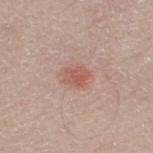Part of a total-body skin-imaging series; this lesion was reviewed on a skin check and was not flagged for biopsy. From the left thigh. Cropped from a total-body skin-imaging series; the visible field is about 15 mm. Approximately 2.5 mm at its widest. A male subject, aged around 70. Captured under white-light illumination.